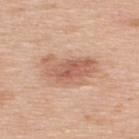Clinical impression: The lesion was photographed on a routine skin check and not biopsied; there is no pathology result. Background: A 15 mm close-up extracted from a 3D total-body photography capture. The total-body-photography lesion software estimated a lesion area of about 16 mm² and a shape eccentricity near 0.85. The analysis additionally found a mean CIELAB color near L≈60 a*≈22 b*≈31 and a lesion–skin lightness drop of about 11. It also reported a nevus-likeness score of about 65/100. Imaged with white-light lighting. A male subject roughly 50 years of age. The lesion's longest dimension is about 6 mm. The lesion is located on the upper back.